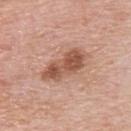This lesion was catalogued during total-body skin photography and was not selected for biopsy. The subject is a male approximately 80 years of age. Imaged with white-light lighting. A 15 mm close-up tile from a total-body photography series done for melanoma screening. The lesion is located on the upper back. Longest diameter approximately 6 mm.